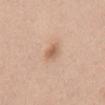Clinical impression: Imaged during a routine full-body skin examination; the lesion was not biopsied and no histopathology is available. Clinical summary: The tile uses white-light illumination. The lesion is located on the mid back. The patient is a female in their 40s. This image is a 15 mm lesion crop taken from a total-body photograph.